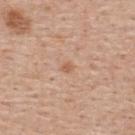Case summary:
– image source — ~15 mm crop, total-body skin-cancer survey
– site — the upper back
– subject — male, roughly 55 years of age